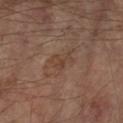Part of a total-body skin-imaging series; this lesion was reviewed on a skin check and was not flagged for biopsy.
Longest diameter approximately 3 mm.
This image is a 15 mm lesion crop taken from a total-body photograph.
A male subject, in their mid-60s.
On the left forearm.
Automated tile analysis of the lesion measured an area of roughly 4 mm², a shape eccentricity near 0.8, and two-axis asymmetry of about 0.6. It also reported a mean CIELAB color near L≈39 a*≈17 b*≈26, about 5 CIELAB-L* units darker than the surrounding skin, and a lesion-to-skin contrast of about 5 (normalized; higher = more distinct). The analysis additionally found an automated nevus-likeness rating near 0 out of 100 and lesion-presence confidence of about 100/100.